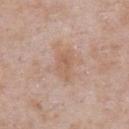workup: imaged on a skin check; not biopsied
automated metrics: an eccentricity of roughly 0.8 and a shape-asymmetry score of about 0.35 (0 = symmetric); an average lesion color of about L≈59 a*≈19 b*≈29 (CIELAB), a lesion–skin lightness drop of about 7, and a normalized border contrast of about 5.5
site: the chest
size: ~3.5 mm (longest diameter)
image: ~15 mm tile from a whole-body skin photo
subject: male, in their mid- to late 50s